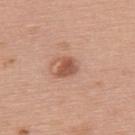notes: total-body-photography surveillance lesion; no biopsy
patient: female, aged 58–62
size: about 2.5 mm
automated metrics: an eccentricity of roughly 0.6 and a shape-asymmetry score of about 0.25 (0 = symmetric)
image source: ~15 mm tile from a whole-body skin photo
site: the upper back
tile lighting: white-light illumination